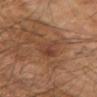Clinical impression:
Imaged during a routine full-body skin examination; the lesion was not biopsied and no histopathology is available.
Background:
The tile uses cross-polarized illumination. The total-body-photography lesion software estimated a lesion area of about 8 mm², a shape eccentricity near 0.85, and a symmetry-axis asymmetry near 0.4. The software also gave a lesion color around L≈41 a*≈21 b*≈29 in CIELAB and a lesion–skin lightness drop of about 6. The patient is a male aged approximately 70. The lesion is on the left forearm. A 15 mm crop from a total-body photograph taken for skin-cancer surveillance. About 5 mm across.